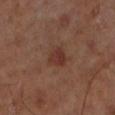Part of a total-body skin-imaging series; this lesion was reviewed on a skin check and was not flagged for biopsy. A close-up tile cropped from a whole-body skin photograph, about 15 mm across. A male subject, aged 68–72. On the leg. The tile uses cross-polarized illumination. About 2.5 mm across.